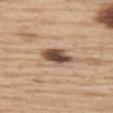Assessment:
Part of a total-body skin-imaging series; this lesion was reviewed on a skin check and was not flagged for biopsy.
Acquisition and patient details:
The lesion is on the left thigh. A 15 mm close-up extracted from a 3D total-body photography capture. Approximately 4 mm at its widest. The lesion-visualizer software estimated a border-irregularity rating of about 2/10, a within-lesion color-variation index near 6.5/10, and a peripheral color-asymmetry measure near 2. And it measured a nevus-likeness score of about 95/100 and a detector confidence of about 100 out of 100 that the crop contains a lesion. Imaged with white-light lighting. A male patient, aged 68–72.